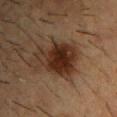Q: What did automated image analysis measure?
A: a mean CIELAB color near L≈24 a*≈16 b*≈24, about 12 CIELAB-L* units darker than the surrounding skin, and a normalized border contrast of about 12.5; a border-irregularity rating of about 3/10 and peripheral color asymmetry of about 1.5
Q: Lesion size?
A: ~5.5 mm (longest diameter)
Q: Lesion location?
A: the upper back
Q: Who is the patient?
A: male, approximately 55 years of age
Q: What kind of image is this?
A: ~15 mm crop, total-body skin-cancer survey
Q: How was the tile lit?
A: cross-polarized illumination